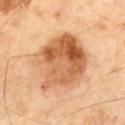Q: Was this lesion biopsied?
A: catalogued during a skin exam; not biopsied
Q: What lighting was used for the tile?
A: cross-polarized
Q: What is the lesion's diameter?
A: ~7.5 mm (longest diameter)
Q: Patient demographics?
A: male, aged around 70
Q: What is the imaging modality?
A: 15 mm crop, total-body photography
Q: Lesion location?
A: the left thigh